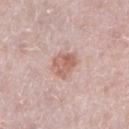workup=total-body-photography surveillance lesion; no biopsy
imaging modality=~15 mm tile from a whole-body skin photo
subject=female, in their 40s
anatomic site=the left lower leg